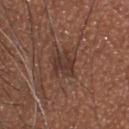Assessment:
Part of a total-body skin-imaging series; this lesion was reviewed on a skin check and was not flagged for biopsy.
Background:
Cropped from a whole-body photographic skin survey; the tile spans about 15 mm. An algorithmic analysis of the crop reported an outline eccentricity of about 0.7 (0 = round, 1 = elongated) and a symmetry-axis asymmetry near 0.3. The analysis additionally found a color-variation rating of about 2/10 and a peripheral color-asymmetry measure near 1. The analysis additionally found lesion-presence confidence of about 95/100. Located on the head or neck. Imaged with white-light lighting. Longest diameter approximately 3.5 mm. The patient is a male roughly 65 years of age.